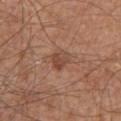notes = catalogued during a skin exam; not biopsied | patient = male, roughly 65 years of age | size = about 2.5 mm | anatomic site = the chest | tile lighting = white-light | automated lesion analysis = border irregularity of about 2 on a 0–10 scale, internal color variation of about 3 on a 0–10 scale, and a peripheral color-asymmetry measure near 1; a nevus-likeness score of about 20/100 and a detector confidence of about 100 out of 100 that the crop contains a lesion | image = 15 mm crop, total-body photography.